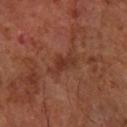Longest diameter approximately 4 mm. A close-up tile cropped from a whole-body skin photograph, about 15 mm across. A male subject, in their 60s. The lesion is on the right forearm. Automated image analysis of the tile measured a lesion color around L≈33 a*≈24 b*≈27 in CIELAB, a lesion–skin lightness drop of about 7, and a lesion-to-skin contrast of about 6.5 (normalized; higher = more distinct). The software also gave a border-irregularity index near 5.5/10, internal color variation of about 2 on a 0–10 scale, and a peripheral color-asymmetry measure near 0.5.